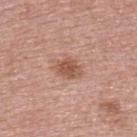Clinical summary:
About 3.5 mm across. From the upper back. A close-up tile cropped from a whole-body skin photograph, about 15 mm across. The subject is a female aged 58 to 62.